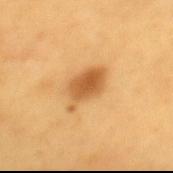Impression: This lesion was catalogued during total-body skin photography and was not selected for biopsy. Acquisition and patient details: An algorithmic analysis of the crop reported an outline eccentricity of about 0.8 (0 = round, 1 = elongated) and a shape-asymmetry score of about 0.3 (0 = symmetric). Imaged with cross-polarized lighting. On the mid back. A roughly 15 mm field-of-view crop from a total-body skin photograph. Longest diameter approximately 4.5 mm. A male patient about 60 years old.